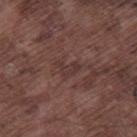Clinical impression:
This lesion was catalogued during total-body skin photography and was not selected for biopsy.
Acquisition and patient details:
This image is a 15 mm lesion crop taken from a total-body photograph. A male patient approximately 75 years of age. Located on the right thigh.